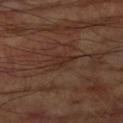| field | value |
|---|---|
| workup | total-body-photography surveillance lesion; no biopsy |
| acquisition | ~15 mm tile from a whole-body skin photo |
| site | the left upper arm |
| patient | male, roughly 60 years of age |